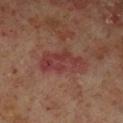workup=catalogued during a skin exam; not biopsied | lighting=cross-polarized | image=~15 mm tile from a whole-body skin photo | location=the left lower leg | size=~6 mm (longest diameter) | patient=male, aged approximately 60.